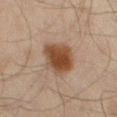Impression: The lesion was photographed on a routine skin check and not biopsied; there is no pathology result. Acquisition and patient details: The patient is a male roughly 45 years of age. A 15 mm close-up tile from a total-body photography series done for melanoma screening. Captured under cross-polarized illumination. Measured at roughly 4 mm in maximum diameter. Automated image analysis of the tile measured an area of roughly 12 mm², an outline eccentricity of about 0.5 (0 = round, 1 = elongated), and a symmetry-axis asymmetry near 0.2. It also reported a lesion color around L≈37 a*≈17 b*≈26 in CIELAB, roughly 11 lightness units darker than nearby skin, and a lesion-to-skin contrast of about 11 (normalized; higher = more distinct). The software also gave a nevus-likeness score of about 100/100 and a lesion-detection confidence of about 100/100. On the left thigh.